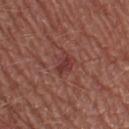Q: Was this lesion biopsied?
A: total-body-photography surveillance lesion; no biopsy
Q: Who is the patient?
A: male, about 55 years old
Q: What is the imaging modality?
A: ~15 mm tile from a whole-body skin photo
Q: What lighting was used for the tile?
A: white-light
Q: Lesion size?
A: ≈2.5 mm
Q: Where on the body is the lesion?
A: the left lower leg